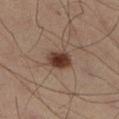Assessment: Imaged during a routine full-body skin examination; the lesion was not biopsied and no histopathology is available. Acquisition and patient details: The lesion is located on the right lower leg. A 15 mm crop from a total-body photograph taken for skin-cancer surveillance. The tile uses cross-polarized illumination. A male patient aged approximately 40. Approximately 3 mm at its widest.